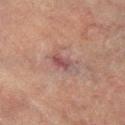A male subject, aged approximately 75. A region of skin cropped from a whole-body photographic capture, roughly 15 mm wide. On the right lower leg. The recorded lesion diameter is about 3 mm. Automated tile analysis of the lesion measured a classifier nevus-likeness of about 0/100. Captured under cross-polarized illumination.